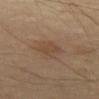Clinical impression: Recorded during total-body skin imaging; not selected for excision or biopsy.